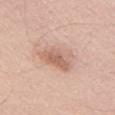biopsy_status: not biopsied; imaged during a skin examination
lighting: white-light
patient:
  sex: male
  age_approx: 55
site: right upper arm
image:
  source: total-body photography crop
  field_of_view_mm: 15
lesion_size:
  long_diameter_mm_approx: 4.5